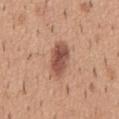| key | value |
|---|---|
| biopsy status | imaged on a skin check; not biopsied |
| TBP lesion metrics | a shape eccentricity near 0.9 and a symmetry-axis asymmetry near 0.15; a lesion color around L≈52 a*≈23 b*≈28 in CIELAB, roughly 14 lightness units darker than nearby skin, and a lesion-to-skin contrast of about 9.5 (normalized; higher = more distinct) |
| patient | male, about 40 years old |
| image | ~15 mm tile from a whole-body skin photo |
| lighting | white-light illumination |
| lesion diameter | ≈5 mm |
| anatomic site | the mid back |